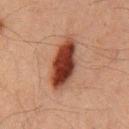Assessment:
Captured during whole-body skin photography for melanoma surveillance; the lesion was not biopsied.
Background:
This is a cross-polarized tile. From the chest. A male subject aged around 60. A close-up tile cropped from a whole-body skin photograph, about 15 mm across. About 7 mm across.